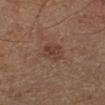{
  "biopsy_status": "not biopsied; imaged during a skin examination",
  "patient": {
    "sex": "male",
    "age_approx": 60
  },
  "site": "left lower leg",
  "image": {
    "source": "total-body photography crop",
    "field_of_view_mm": 15
  }
}